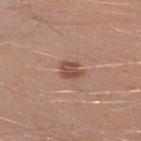Notes:
– follow-up — imaged on a skin check; not biopsied
– imaging modality — ~15 mm crop, total-body skin-cancer survey
– patient — male, aged 28 to 32
– body site — the leg
– automated lesion analysis — a lesion area of about 4 mm², an outline eccentricity of about 0.65 (0 = round, 1 = elongated), and a shape-asymmetry score of about 0.3 (0 = symmetric); border irregularity of about 3 on a 0–10 scale, a within-lesion color-variation index near 4/10, and peripheral color asymmetry of about 1.5; a classifier nevus-likeness of about 45/100 and a lesion-detection confidence of about 100/100